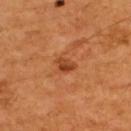workup = imaged on a skin check; not biopsied
image = ~15 mm crop, total-body skin-cancer survey
subject = male, in their mid- to late 50s
automated lesion analysis = border irregularity of about 2.5 on a 0–10 scale, a color-variation rating of about 3.5/10, and a peripheral color-asymmetry measure near 1.5
lesion diameter = about 2.5 mm
anatomic site = the upper back